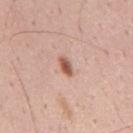follow-up = no biopsy performed (imaged during a skin exam); patient = male, approximately 50 years of age; diameter = ≈3 mm; tile lighting = white-light; image source = 15 mm crop, total-body photography; anatomic site = the mid back.